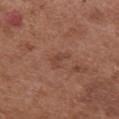• notes · imaged on a skin check; not biopsied
• patient · female, about 75 years old
• imaging modality · ~15 mm crop, total-body skin-cancer survey
• site · the chest
• tile lighting · white-light illumination
• size · ≈2.5 mm
• TBP lesion metrics · an area of roughly 2.5 mm², an outline eccentricity of about 0.85 (0 = round, 1 = elongated), and two-axis asymmetry of about 0.5; an average lesion color of about L≈43 a*≈22 b*≈27 (CIELAB) and a normalized lesion–skin contrast near 5